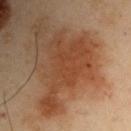biopsy status = no biopsy performed (imaged during a skin exam) | image source = ~15 mm tile from a whole-body skin photo | body site = the left upper arm | automated lesion analysis = a border-irregularity rating of about 9.5/10, internal color variation of about 2.5 on a 0–10 scale, and peripheral color asymmetry of about 1 | patient = male, in their mid-50s.